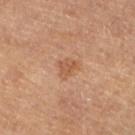follow-up = imaged on a skin check; not biopsied
lesion size = ≈2.5 mm
imaging modality = total-body-photography crop, ~15 mm field of view
subject = female, aged around 60
tile lighting = cross-polarized illumination
location = the left lower leg